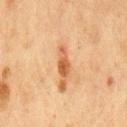Case summary:
- follow-up: imaged on a skin check; not biopsied
- subject: male, aged around 75
- image: ~15 mm tile from a whole-body skin photo
- site: the front of the torso